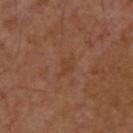Context: On the upper back. Captured under cross-polarized illumination. A male patient aged 53 to 57. Approximately 3 mm at its widest. An algorithmic analysis of the crop reported a shape eccentricity near 0.8 and a symmetry-axis asymmetry near 0.35. It also reported a lesion color around L≈39 a*≈21 b*≈30 in CIELAB, a lesion–skin lightness drop of about 4, and a lesion-to-skin contrast of about 4.5 (normalized; higher = more distinct). It also reported border irregularity of about 4 on a 0–10 scale, internal color variation of about 1 on a 0–10 scale, and radial color variation of about 0.5. The software also gave a classifier nevus-likeness of about 0/100 and a lesion-detection confidence of about 100/100. This image is a 15 mm lesion crop taken from a total-body photograph.